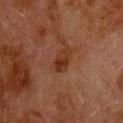The lesion was tiled from a total-body skin photograph and was not biopsied.
About 3 mm across.
This is a cross-polarized tile.
From the back.
A male patient, about 80 years old.
A 15 mm close-up tile from a total-body photography series done for melanoma screening.